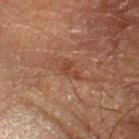<case>
  <automated_metrics>
    <border_irregularity_0_10>5.0</border_irregularity_0_10>
    <color_variation_0_10>1.0</color_variation_0_10>
    <peripheral_color_asymmetry>0.5</peripheral_color_asymmetry>
  </automated_metrics>
  <site>left forearm</site>
  <patient>
    <sex>male</sex>
    <age_approx>65</age_approx>
  </patient>
  <image>
    <source>total-body photography crop</source>
    <field_of_view_mm>15</field_of_view_mm>
  </image>
  <lighting>cross-polarized</lighting>
</case>